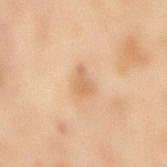<record>
  <lesion_size>
    <long_diameter_mm_approx>2.5</long_diameter_mm_approx>
  </lesion_size>
  <image>
    <source>total-body photography crop</source>
    <field_of_view_mm>15</field_of_view_mm>
  </image>
  <patient>
    <sex>female</sex>
    <age_approx>55</age_approx>
  </patient>
  <site>mid back</site>
  <lighting>cross-polarized</lighting>
</record>